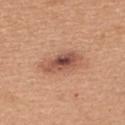notes — imaged on a skin check; not biopsied | acquisition — ~15 mm tile from a whole-body skin photo | site — the upper back | TBP lesion metrics — border irregularity of about 2.5 on a 0–10 scale, a within-lesion color-variation index near 8/10, and radial color variation of about 2.5; a classifier nevus-likeness of about 90/100 and a lesion-detection confidence of about 100/100 | patient — female, in their 30s | tile lighting — white-light.